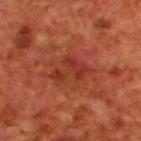No biopsy was performed on this lesion — it was imaged during a full skin examination and was not determined to be concerning. From the upper back. Automated image analysis of the tile measured a lesion color around L≈36 a*≈33 b*≈33 in CIELAB and a normalized lesion–skin contrast near 6. Cropped from a whole-body photographic skin survey; the tile spans about 15 mm. About 4.5 mm across. A male patient aged around 70. Imaged with cross-polarized lighting.